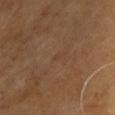Part of a total-body skin-imaging series; this lesion was reviewed on a skin check and was not flagged for biopsy.
Automated tile analysis of the lesion measured a lesion area of about 1 mm², a shape eccentricity near 0.7, and two-axis asymmetry of about 0.25. The analysis additionally found a border-irregularity index near 2/10, internal color variation of about 0 on a 0–10 scale, and radial color variation of about 0.
A region of skin cropped from a whole-body photographic capture, roughly 15 mm wide.
This is a cross-polarized tile.
A male patient roughly 65 years of age.
The lesion is located on the chest.
The recorded lesion diameter is about 1 mm.